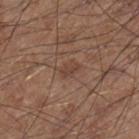Part of a total-body skin-imaging series; this lesion was reviewed on a skin check and was not flagged for biopsy. A male subject about 60 years old. Cropped from a whole-body photographic skin survey; the tile spans about 15 mm. From the right lower leg. The recorded lesion diameter is about 2.5 mm. An algorithmic analysis of the crop reported border irregularity of about 2.5 on a 0–10 scale, a color-variation rating of about 2/10, and peripheral color asymmetry of about 1. And it measured a classifier nevus-likeness of about 5/100. Captured under white-light illumination.